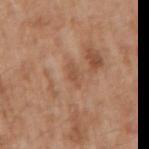Imaged during a routine full-body skin examination; the lesion was not biopsied and no histopathology is available.
The patient is a male aged 63 to 67.
The lesion is on the arm.
Longest diameter approximately 3 mm.
A 15 mm close-up tile from a total-body photography series done for melanoma screening.
An algorithmic analysis of the crop reported a lesion area of about 3 mm² and an eccentricity of roughly 0.9. It also reported a mean CIELAB color near L≈52 a*≈21 b*≈32. And it measured a border-irregularity index near 3/10, internal color variation of about 1 on a 0–10 scale, and peripheral color asymmetry of about 0.5. The analysis additionally found a nevus-likeness score of about 0/100 and lesion-presence confidence of about 100/100.
The tile uses white-light illumination.